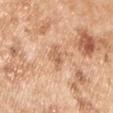Impression: No biopsy was performed on this lesion — it was imaged during a full skin examination and was not determined to be concerning. Background: The lesion-visualizer software estimated a mean CIELAB color near L≈63 a*≈22 b*≈34, a lesion–skin lightness drop of about 9, and a lesion-to-skin contrast of about 6 (normalized; higher = more distinct). The software also gave a classifier nevus-likeness of about 0/100. A lesion tile, about 15 mm wide, cut from a 3D total-body photograph. Captured under white-light illumination. From the left upper arm. A male subject in their mid- to late 50s.